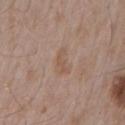Clinical impression:
Recorded during total-body skin imaging; not selected for excision or biopsy.
Background:
A male patient, aged 53 to 57. From the right upper arm. A 15 mm close-up extracted from a 3D total-body photography capture. Automated image analysis of the tile measured a footprint of about 4.5 mm² and an outline eccentricity of about 0.9 (0 = round, 1 = elongated). And it measured an average lesion color of about L≈54 a*≈17 b*≈27 (CIELAB), about 6 CIELAB-L* units darker than the surrounding skin, and a lesion-to-skin contrast of about 5 (normalized; higher = more distinct). The analysis additionally found a border-irregularity index near 4.5/10, a color-variation rating of about 0.5/10, and peripheral color asymmetry of about 0. And it measured an automated nevus-likeness rating near 0 out of 100 and lesion-presence confidence of about 100/100.